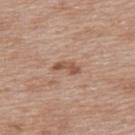Clinical impression:
This lesion was catalogued during total-body skin photography and was not selected for biopsy.
Image and clinical context:
A female subject, aged approximately 40. A region of skin cropped from a whole-body photographic capture, roughly 15 mm wide. From the upper back. Automated image analysis of the tile measured an outline eccentricity of about 0.9 (0 = round, 1 = elongated) and a shape-asymmetry score of about 0.35 (0 = symmetric). It also reported a lesion color around L≈53 a*≈20 b*≈30 in CIELAB, about 11 CIELAB-L* units darker than the surrounding skin, and a normalized lesion–skin contrast near 7.5. And it measured a within-lesion color-variation index near 0.5/10 and radial color variation of about 0. The analysis additionally found an automated nevus-likeness rating near 5 out of 100. The lesion's longest dimension is about 3 mm.